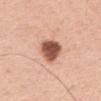  biopsy_status: not biopsied; imaged during a skin examination
  image:
    source: total-body photography crop
    field_of_view_mm: 15
  patient:
    sex: male
    age_approx: 60
  site: mid back
  lighting: white-light
  lesion_size:
    long_diameter_mm_approx: 4.5